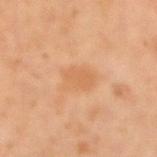biopsy status — catalogued during a skin exam; not biopsied | size — ~3.5 mm (longest diameter) | image — 15 mm crop, total-body photography | patient — male, in their 60s | site — the right upper arm.